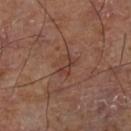This lesion was catalogued during total-body skin photography and was not selected for biopsy.
This is a cross-polarized tile.
The recorded lesion diameter is about 2.5 mm.
From the leg.
The total-body-photography lesion software estimated a border-irregularity rating of about 2.5/10 and peripheral color asymmetry of about 1.5. The software also gave a classifier nevus-likeness of about 0/100.
Cropped from a whole-body photographic skin survey; the tile spans about 15 mm.